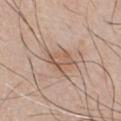<record>
  <biopsy_status>not biopsied; imaged during a skin examination</biopsy_status>
  <patient>
    <sex>male</sex>
    <age_approx>45</age_approx>
  </patient>
  <image>
    <source>total-body photography crop</source>
    <field_of_view_mm>15</field_of_view_mm>
  </image>
  <site>front of the torso</site>
  <lighting>white-light</lighting>
  <lesion_size>
    <long_diameter_mm_approx>3.0</long_diameter_mm_approx>
  </lesion_size>
  <automated_metrics>
    <border_irregularity_0_10>3.5</border_irregularity_0_10>
    <color_variation_0_10>5.0</color_variation_0_10>
    <peripheral_color_asymmetry>1.5</peripheral_color_asymmetry>
  </automated_metrics>
</record>